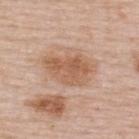A lesion tile, about 15 mm wide, cut from a 3D total-body photograph. Located on the upper back. Longest diameter approximately 5.5 mm. Captured under white-light illumination. A female patient aged around 50.